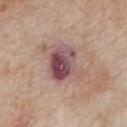Q: Is there a histopathology result?
A: imaged on a skin check; not biopsied
Q: What is the anatomic site?
A: the chest
Q: What kind of image is this?
A: 15 mm crop, total-body photography
Q: Who is the patient?
A: male, aged around 65
Q: How was the tile lit?
A: white-light